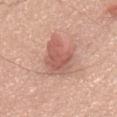Findings:
- body site: the upper back
- diameter: ~5.5 mm (longest diameter)
- image: 15 mm crop, total-body photography
- patient: male, aged 73–77
- tile lighting: white-light illumination